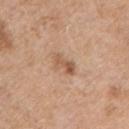| feature | finding |
|---|---|
| biopsy status | no biopsy performed (imaged during a skin exam) |
| location | the chest |
| imaging modality | 15 mm crop, total-body photography |
| TBP lesion metrics | roughly 10 lightness units darker than nearby skin; a border-irregularity index near 4/10 and a peripheral color-asymmetry measure near 2.5; a nevus-likeness score of about 10/100 and lesion-presence confidence of about 100/100 |
| illumination | white-light illumination |
| patient | male, roughly 55 years of age |